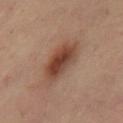A female subject aged 38–42. Longest diameter approximately 6 mm. A 15 mm crop from a total-body photograph taken for skin-cancer surveillance. This is a cross-polarized tile. The lesion is located on the right thigh. Automated tile analysis of the lesion measured a mean CIELAB color near L≈44 a*≈21 b*≈28, about 12 CIELAB-L* units darker than the surrounding skin, and a normalized lesion–skin contrast near 9. The analysis additionally found a color-variation rating of about 6/10 and radial color variation of about 1.5.